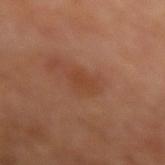Impression:
No biopsy was performed on this lesion — it was imaged during a full skin examination and was not determined to be concerning.
Context:
A female patient in their mid-50s. A lesion tile, about 15 mm wide, cut from a 3D total-body photograph. From the arm.